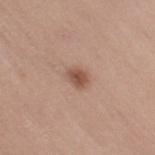Q: Was this lesion biopsied?
A: total-body-photography surveillance lesion; no biopsy
Q: What are the patient's age and sex?
A: female, aged approximately 45
Q: Where on the body is the lesion?
A: the back
Q: What kind of image is this?
A: ~15 mm tile from a whole-body skin photo
Q: What did automated image analysis measure?
A: an area of roughly 3.5 mm²; an average lesion color of about L≈52 a*≈21 b*≈28 (CIELAB); a border-irregularity rating of about 2/10 and a color-variation rating of about 3/10; a classifier nevus-likeness of about 95/100 and a detector confidence of about 100 out of 100 that the crop contains a lesion
Q: How was the tile lit?
A: white-light illumination